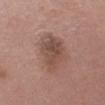Part of a total-body skin-imaging series; this lesion was reviewed on a skin check and was not flagged for biopsy. The lesion is on the right thigh. Imaged with white-light lighting. A female patient, about 50 years old. About 6 mm across. Cropped from a total-body skin-imaging series; the visible field is about 15 mm.